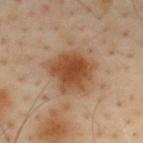This lesion was catalogued during total-body skin photography and was not selected for biopsy. A lesion tile, about 15 mm wide, cut from a 3D total-body photograph. Located on the upper back. Imaged with cross-polarized lighting. Measured at roughly 6 mm in maximum diameter. The subject is a male aged around 55.